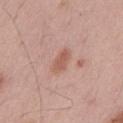{"image": {"source": "total-body photography crop", "field_of_view_mm": 15}, "lighting": "white-light", "automated_metrics": {"area_mm2_approx": 4.5, "eccentricity": 0.85, "shape_asymmetry": 0.3, "cielab_L": 57, "cielab_a": 22, "cielab_b": 28, "color_variation_0_10": 1.5, "peripheral_color_asymmetry": 0.5, "nevus_likeness_0_100": 25, "lesion_detection_confidence_0_100": 100}, "lesion_size": {"long_diameter_mm_approx": 3.0}, "site": "mid back", "patient": {"sex": "male", "age_approx": 55}}